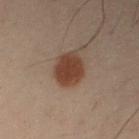biopsy status: no biopsy performed (imaged during a skin exam) | lighting: cross-polarized illumination | subject: male, about 50 years old | imaging modality: ~15 mm crop, total-body skin-cancer survey | site: the left arm | image-analysis metrics: a mean CIELAB color near L≈31 a*≈17 b*≈23, about 11 CIELAB-L* units darker than the surrounding skin, and a lesion-to-skin contrast of about 10.5 (normalized; higher = more distinct); a classifier nevus-likeness of about 100/100 and a lesion-detection confidence of about 100/100.